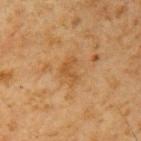subject=male, aged approximately 60 | imaging modality=15 mm crop, total-body photography | body site=the left upper arm.